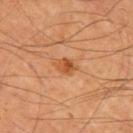automated_metrics:
  nevus_likeness_0_100: 75
  lesion_detection_confidence_0_100: 100
site: mid back
patient:
  sex: male
  age_approx: 55
image:
  source: total-body photography crop
  field_of_view_mm: 15